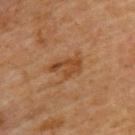| key | value |
|---|---|
| follow-up | catalogued during a skin exam; not biopsied |
| anatomic site | the upper back |
| image source | ~15 mm tile from a whole-body skin photo |
| illumination | cross-polarized illumination |
| patient | male, approximately 60 years of age |
| diameter | about 4 mm |